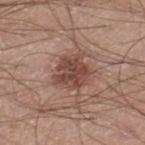biopsy_status: not biopsied; imaged during a skin examination
automated_metrics:
  area_mm2_approx: 11.0
  eccentricity: 0.3
  shape_asymmetry: 0.2
  cielab_L: 45
  cielab_a: 21
  cielab_b: 24
  vs_skin_darker_L: 11.0
  vs_skin_contrast_norm: 8.5
  border_irregularity_0_10: 2.5
  peripheral_color_asymmetry: 1.5
  lesion_detection_confidence_0_100: 100
patient:
  sex: male
  age_approx: 20
image:
  source: total-body photography crop
  field_of_view_mm: 15
lesion_size:
  long_diameter_mm_approx: 4.0
lighting: white-light
site: left thigh